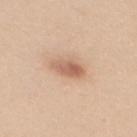Captured during whole-body skin photography for melanoma surveillance; the lesion was not biopsied.
Imaged with white-light lighting.
The lesion is on the mid back.
A female subject, aged 43 to 47.
About 3.5 mm across.
Cropped from a total-body skin-imaging series; the visible field is about 15 mm.